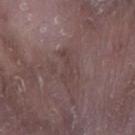The lesion was tiled from a total-body skin photograph and was not biopsied. Automated tile analysis of the lesion measured an average lesion color of about L≈40 a*≈16 b*≈17 (CIELAB), roughly 5 lightness units darker than nearby skin, and a normalized lesion–skin contrast near 4.5. And it measured an automated nevus-likeness rating near 0 out of 100. A male subject aged 73 to 77. Approximately 3.5 mm at its widest. Captured under white-light illumination. On the right lower leg. A region of skin cropped from a whole-body photographic capture, roughly 15 mm wide.